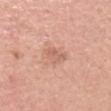The lesion was tiled from a total-body skin photograph and was not biopsied. A female patient about 65 years old. Cropped from a whole-body photographic skin survey; the tile spans about 15 mm. Located on the head or neck.